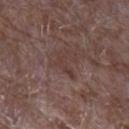Notes:
• workup — total-body-photography surveillance lesion; no biopsy
• image source — 15 mm crop, total-body photography
• tile lighting — white-light
• location — the left forearm
• patient — male, in their mid-60s
• size — about 3.5 mm